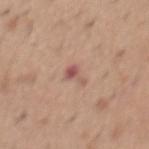The subject is a male roughly 40 years of age.
A lesion tile, about 15 mm wide, cut from a 3D total-body photograph.
Automated tile analysis of the lesion measured an eccentricity of roughly 0.9 and a shape-asymmetry score of about 0.55 (0 = symmetric). And it measured an average lesion color of about L≈54 a*≈23 b*≈24 (CIELAB), about 10 CIELAB-L* units darker than the surrounding skin, and a normalized border contrast of about 7.5. And it measured border irregularity of about 6 on a 0–10 scale, a color-variation rating of about 0.5/10, and peripheral color asymmetry of about 0.
On the mid back.